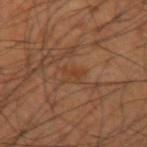Assessment: Captured during whole-body skin photography for melanoma surveillance; the lesion was not biopsied. Image and clinical context: Located on the left upper arm. A male patient in their 40s. The lesion's longest dimension is about 2.5 mm. A roughly 15 mm field-of-view crop from a total-body skin photograph.